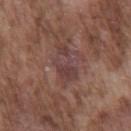lighting: white-light | subject: male, aged around 75 | acquisition: total-body-photography crop, ~15 mm field of view | lesion size: about 4.5 mm | body site: the chest.